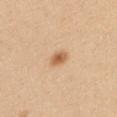Clinical impression:
This lesion was catalogued during total-body skin photography and was not selected for biopsy.
Acquisition and patient details:
On the mid back. The subject is a female in their mid- to late 20s. Cropped from a total-body skin-imaging series; the visible field is about 15 mm.